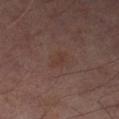Q: Was this lesion biopsied?
A: total-body-photography surveillance lesion; no biopsy
Q: What are the patient's age and sex?
A: male, aged 63 to 67
Q: What kind of image is this?
A: ~15 mm tile from a whole-body skin photo
Q: What is the lesion's diameter?
A: about 2.5 mm
Q: Where on the body is the lesion?
A: the left thigh